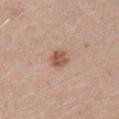– workup — no biopsy performed (imaged during a skin exam)
– automated lesion analysis — a lesion color around L≈54 a*≈21 b*≈29 in CIELAB, about 12 CIELAB-L* units darker than the surrounding skin, and a lesion-to-skin contrast of about 8 (normalized; higher = more distinct)
– image — total-body-photography crop, ~15 mm field of view
– patient — male, aged 23–27
– diameter — about 2.5 mm
– tile lighting — white-light illumination
– location — the right upper arm